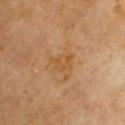Q: Who is the patient?
A: male, roughly 60 years of age
Q: What kind of image is this?
A: total-body-photography crop, ~15 mm field of view
Q: How large is the lesion?
A: ~3 mm (longest diameter)
Q: Illumination type?
A: cross-polarized illumination
Q: Automated lesion metrics?
A: a footprint of about 5 mm², a shape eccentricity near 0.65, and two-axis asymmetry of about 0.35; a border-irregularity rating of about 3.5/10 and a within-lesion color-variation index near 1.5/10
Q: Where on the body is the lesion?
A: the right arm